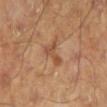  biopsy_status: not biopsied; imaged during a skin examination
  site: left lower leg
  image:
    source: total-body photography crop
    field_of_view_mm: 15
  patient:
    sex: male
    age_approx: 45
  lighting: cross-polarized
  automated_metrics:
    area_mm2_approx: 5.0
    shape_asymmetry: 0.65
    cielab_L: 50
    cielab_a: 22
    cielab_b: 33
    vs_skin_contrast_norm: 6.0
    lesion_detection_confidence_0_100: 100